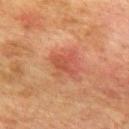Impression: This lesion was catalogued during total-body skin photography and was not selected for biopsy. Clinical summary: The subject is a male aged 73 to 77. Measured at roughly 3 mm in maximum diameter. A region of skin cropped from a whole-body photographic capture, roughly 15 mm wide. Located on the mid back.